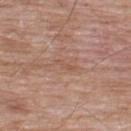Imaged during a routine full-body skin examination; the lesion was not biopsied and no histopathology is available. The patient is a male approximately 60 years of age. A 15 mm close-up extracted from a 3D total-body photography capture. Measured at roughly 2.5 mm in maximum diameter. From the upper back. Imaged with white-light lighting.